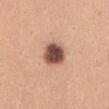Q: What did automated image analysis measure?
A: a lesion area of about 8.5 mm²; a border-irregularity index near 1.5/10, a within-lesion color-variation index near 5.5/10, and a peripheral color-asymmetry measure near 1.5; an automated nevus-likeness rating near 65 out of 100 and a detector confidence of about 100 out of 100 that the crop contains a lesion
Q: How was the tile lit?
A: white-light
Q: Patient demographics?
A: female, aged around 30
Q: What is the imaging modality?
A: 15 mm crop, total-body photography
Q: Where on the body is the lesion?
A: the abdomen
Q: Lesion size?
A: ≈3.5 mm